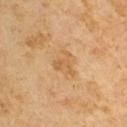workup = no biopsy performed (imaged during a skin exam) | lesion diameter = about 3 mm | subject = male, about 65 years old | image = total-body-photography crop, ~15 mm field of view | image-analysis metrics = a footprint of about 3.5 mm², an eccentricity of roughly 0.3, and a symmetry-axis asymmetry near 0.6; an average lesion color of about L≈58 a*≈19 b*≈41 (CIELAB) and a normalized lesion–skin contrast near 5.5; a classifier nevus-likeness of about 0/100.